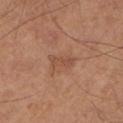biopsy status: no biopsy performed (imaged during a skin exam) | image: ~15 mm tile from a whole-body skin photo | tile lighting: cross-polarized | site: the left lower leg | lesion size: ≈3 mm | patient: male, in their 60s.